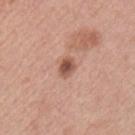imaging modality: total-body-photography crop, ~15 mm field of view; subject: male, roughly 60 years of age; lighting: white-light; lesion size: about 2.5 mm; site: the chest.